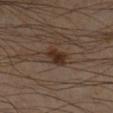Recorded during total-body skin imaging; not selected for excision or biopsy.
A male subject, aged 43 to 47.
A region of skin cropped from a whole-body photographic capture, roughly 15 mm wide.
From the left forearm.
Imaged with cross-polarized lighting.
Longest diameter approximately 2.5 mm.